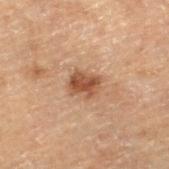The lesion was photographed on a routine skin check and not biopsied; there is no pathology result.
A 15 mm crop from a total-body photograph taken for skin-cancer surveillance.
A male subject aged approximately 70.
An algorithmic analysis of the crop reported a lesion area of about 6 mm², a shape eccentricity near 0.65, and a symmetry-axis asymmetry near 0.2. The software also gave a mean CIELAB color near L≈38 a*≈18 b*≈26, roughly 10 lightness units darker than nearby skin, and a normalized lesion–skin contrast near 9. And it measured lesion-presence confidence of about 100/100.
Located on the leg.
Approximately 3 mm at its widest.